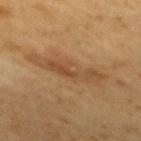{"biopsy_status": "not biopsied; imaged during a skin examination", "patient": {"sex": "male", "age_approx": 60}, "image": {"source": "total-body photography crop", "field_of_view_mm": 15}, "site": "mid back"}